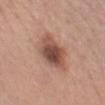Located on the chest. A female subject, in their mid-50s. A 15 mm crop from a total-body photograph taken for skin-cancer surveillance.